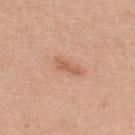Recorded during total-body skin imaging; not selected for excision or biopsy. A female patient, roughly 40 years of age. A lesion tile, about 15 mm wide, cut from a 3D total-body photograph. The lesion is located on the back.